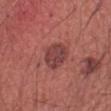biopsy status: catalogued during a skin exam; not biopsied
diameter: about 3.5 mm
TBP lesion metrics: a border-irregularity rating of about 1.5/10, internal color variation of about 3.5 on a 0–10 scale, and a peripheral color-asymmetry measure near 1.5; an automated nevus-likeness rating near 95 out of 100 and a lesion-detection confidence of about 100/100
tile lighting: white-light
site: the abdomen
patient: male, roughly 65 years of age
acquisition: 15 mm crop, total-body photography